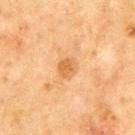imaging modality: ~15 mm tile from a whole-body skin photo
lighting: cross-polarized
patient: male, aged around 65
body site: the chest